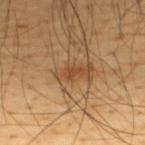<lesion>
<biopsy_status>not biopsied; imaged during a skin examination</biopsy_status>
<automated_metrics>
  <area_mm2_approx>2.0</area_mm2_approx>
  <eccentricity>0.8</eccentricity>
  <shape_asymmetry>0.35</shape_asymmetry>
  <cielab_L>44</cielab_L>
  <cielab_a>22</cielab_a>
  <cielab_b>35</cielab_b>
  <vs_skin_darker_L>8.0</vs_skin_darker_L>
  <vs_skin_contrast_norm>6.5</vs_skin_contrast_norm>
  <border_irregularity_0_10>3.5</border_irregularity_0_10>
  <color_variation_0_10>0.0</color_variation_0_10>
  <peripheral_color_asymmetry>0.0</peripheral_color_asymmetry>
  <nevus_likeness_0_100>45</nevus_likeness_0_100>
  <lesion_detection_confidence_0_100>95</lesion_detection_confidence_0_100>
</automated_metrics>
<site>upper back</site>
<lighting>cross-polarized</lighting>
<image>
  <source>total-body photography crop</source>
  <field_of_view_mm>15</field_of_view_mm>
</image>
<lesion_size>
  <long_diameter_mm_approx>2.0</long_diameter_mm_approx>
</lesion_size>
<patient>
  <sex>male</sex>
  <age_approx>45</age_approx>
</patient>
</lesion>